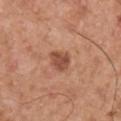Q: Was this lesion biopsied?
A: total-body-photography surveillance lesion; no biopsy